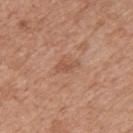| field | value |
|---|---|
| biopsy status | total-body-photography surveillance lesion; no biopsy |
| lesion diameter | ≈2.5 mm |
| subject | male, aged around 80 |
| image | ~15 mm crop, total-body skin-cancer survey |
| location | the upper back |